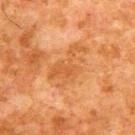Imaged with cross-polarized lighting. Automated tile analysis of the lesion measured an area of roughly 12 mm², a shape eccentricity near 0.85, and a shape-asymmetry score of about 0.55 (0 = symmetric). On the upper back. This image is a 15 mm lesion crop taken from a total-body photograph. A male patient aged 78–82. The lesion's longest dimension is about 5.5 mm.